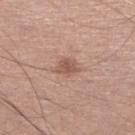Part of a total-body skin-imaging series; this lesion was reviewed on a skin check and was not flagged for biopsy. On the leg. A male patient aged approximately 55. A roughly 15 mm field-of-view crop from a total-body skin photograph.